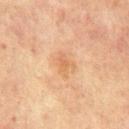Q: Is there a histopathology result?
A: no biopsy performed (imaged during a skin exam)
Q: Lesion location?
A: the abdomen
Q: How large is the lesion?
A: ≈2.5 mm
Q: What is the imaging modality?
A: ~15 mm crop, total-body skin-cancer survey
Q: Who is the patient?
A: male, in their mid- to late 60s
Q: What lighting was used for the tile?
A: cross-polarized